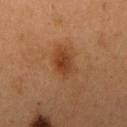Assessment: Captured during whole-body skin photography for melanoma surveillance; the lesion was not biopsied. Acquisition and patient details: On the right upper arm. A 15 mm close-up tile from a total-body photography series done for melanoma screening. Imaged with cross-polarized lighting. Approximately 4 mm at its widest. A female subject aged around 40.